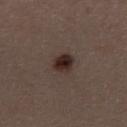follow-up: imaged on a skin check; not biopsied | automated lesion analysis: an area of roughly 5 mm², an eccentricity of roughly 0.45, and a shape-asymmetry score of about 0.2 (0 = symmetric); internal color variation of about 5 on a 0–10 scale and radial color variation of about 1.5; an automated nevus-likeness rating near 100 out of 100 and a lesion-detection confidence of about 100/100 | patient: female, roughly 30 years of age | site: the upper back | acquisition: 15 mm crop, total-body photography | diameter: ≈2.5 mm | lighting: white-light illumination.